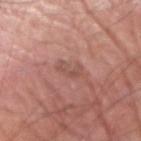The lesion was tiled from a total-body skin photograph and was not biopsied.
From the arm.
A 15 mm crop from a total-body photograph taken for skin-cancer surveillance.
A male subject roughly 75 years of age.
Longest diameter approximately 3 mm.
The tile uses white-light illumination.